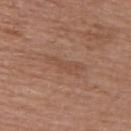Q: Was this lesion biopsied?
A: no biopsy performed (imaged during a skin exam)
Q: What are the patient's age and sex?
A: female, in their 60s
Q: How was this image acquired?
A: ~15 mm tile from a whole-body skin photo
Q: Lesion location?
A: the back
Q: What did automated image analysis measure?
A: a lesion area of about 6 mm², a shape eccentricity near 0.95, and two-axis asymmetry of about 0.4
Q: What is the lesion's diameter?
A: ~5 mm (longest diameter)